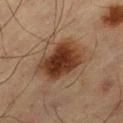| feature | finding |
|---|---|
| biopsy status | total-body-photography surveillance lesion; no biopsy |
| anatomic site | the right thigh |
| imaging modality | 15 mm crop, total-body photography |
| patient | male, in their mid-60s |